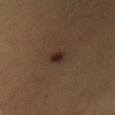Recorded during total-body skin imaging; not selected for excision or biopsy.
The lesion is on the chest.
The recorded lesion diameter is about 3 mm.
A female patient about 30 years old.
A 15 mm close-up tile from a total-body photography series done for melanoma screening.